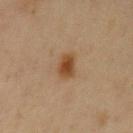Recorded during total-body skin imaging; not selected for excision or biopsy. Measured at roughly 3 mm in maximum diameter. On the left upper arm. A close-up tile cropped from a whole-body skin photograph, about 15 mm across. The subject is a female approximately 45 years of age.